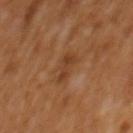Notes:
- follow-up · catalogued during a skin exam; not biopsied
- image · ~15 mm crop, total-body skin-cancer survey
- lighting · cross-polarized
- subject · male, aged 63–67
- size · ~3 mm (longest diameter)
- site · the mid back